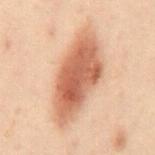notes: imaged on a skin check; not biopsied | location: the mid back | image: 15 mm crop, total-body photography | subject: male, about 50 years old | illumination: cross-polarized | lesion size: about 10.5 mm.